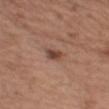Assessment: Part of a total-body skin-imaging series; this lesion was reviewed on a skin check and was not flagged for biopsy. Background: A region of skin cropped from a whole-body photographic capture, roughly 15 mm wide. A male subject, approximately 55 years of age. Located on the left upper arm. Longest diameter approximately 2.5 mm. Automated tile analysis of the lesion measured a lesion color around L≈44 a*≈20 b*≈26 in CIELAB, roughly 11 lightness units darker than nearby skin, and a lesion-to-skin contrast of about 8.5 (normalized; higher = more distinct).